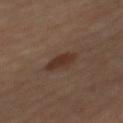Imaged during a routine full-body skin examination; the lesion was not biopsied and no histopathology is available. From the mid back. A region of skin cropped from a whole-body photographic capture, roughly 15 mm wide. The subject is a male approximately 70 years of age.